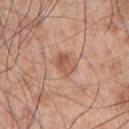Part of a total-body skin-imaging series; this lesion was reviewed on a skin check and was not flagged for biopsy.
The lesion-visualizer software estimated an area of roughly 4.5 mm², an eccentricity of roughly 0.75, and a symmetry-axis asymmetry near 0.3. The analysis additionally found a border-irregularity rating of about 3/10, a within-lesion color-variation index near 4.5/10, and a peripheral color-asymmetry measure near 2.
The subject is a male approximately 70 years of age.
Cropped from a total-body skin-imaging series; the visible field is about 15 mm.
Imaged with white-light lighting.
The lesion is on the arm.
About 3 mm across.